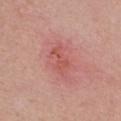Clinical impression: The lesion was photographed on a routine skin check and not biopsied; there is no pathology result. Background: Automated tile analysis of the lesion measured a lesion area of about 7 mm², a shape eccentricity near 0.8, and a shape-asymmetry score of about 0.3 (0 = symmetric). It also reported a border-irregularity rating of about 4.5/10 and a peripheral color-asymmetry measure near 1. A 15 mm close-up tile from a total-body photography series done for melanoma screening. A female patient aged around 25. This is a white-light tile. From the chest.